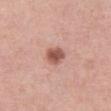Impression: The lesion was photographed on a routine skin check and not biopsied; there is no pathology result. Acquisition and patient details: A roughly 15 mm field-of-view crop from a total-body skin photograph. Measured at roughly 3 mm in maximum diameter. The subject is a female aged 38 to 42. Located on the right thigh.